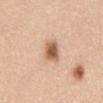• biopsy status — catalogued during a skin exam; not biopsied
• location — the abdomen
• lesion size — about 3 mm
• acquisition — ~15 mm tile from a whole-body skin photo
• illumination — white-light
• subject — male, aged around 60